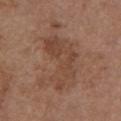This is a white-light tile.
A female patient, in their mid- to late 60s.
Located on the front of the torso.
Cropped from a whole-body photographic skin survey; the tile spans about 15 mm.